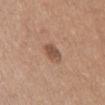• biopsy status · catalogued during a skin exam; not biopsied
• lesion diameter · ≈2.5 mm
• automated metrics · a mean CIELAB color near L≈51 a*≈20 b*≈28, a lesion–skin lightness drop of about 11, and a lesion-to-skin contrast of about 8 (normalized; higher = more distinct); a nevus-likeness score of about 45/100 and a detector confidence of about 100 out of 100 that the crop contains a lesion
• image · total-body-photography crop, ~15 mm field of view
• patient · female, aged 43 to 47
• anatomic site · the abdomen
• tile lighting · white-light illumination